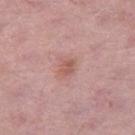Q: Was a biopsy performed?
A: no biopsy performed (imaged during a skin exam)
Q: What lighting was used for the tile?
A: white-light
Q: How was this image acquired?
A: 15 mm crop, total-body photography
Q: What is the anatomic site?
A: the right thigh
Q: What is the lesion's diameter?
A: ≈2.5 mm
Q: Patient demographics?
A: female, in their 60s